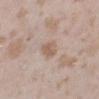biopsy_status: not biopsied; imaged during a skin examination
patient:
  sex: female
  age_approx: 25
image:
  source: total-body photography crop
  field_of_view_mm: 15
site: left lower leg